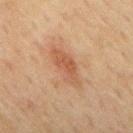Assessment:
The lesion was tiled from a total-body skin photograph and was not biopsied.
Image and clinical context:
A male subject in their 70s. The lesion is on the mid back. Automated image analysis of the tile measured a footprint of about 10 mm², an outline eccentricity of about 0.9 (0 = round, 1 = elongated), and two-axis asymmetry of about 0.25. The analysis additionally found a mean CIELAB color near L≈46 a*≈19 b*≈29 and a normalized border contrast of about 6. It also reported a peripheral color-asymmetry measure near 1. A 15 mm close-up tile from a total-body photography series done for melanoma screening. The tile uses cross-polarized illumination. Measured at roughly 5.5 mm in maximum diameter.